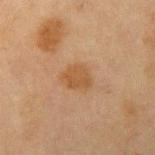workup: total-body-photography surveillance lesion; no biopsy
lesion size: ≈3 mm
image source: total-body-photography crop, ~15 mm field of view
subject: male, in their mid- to late 60s
TBP lesion metrics: an area of roughly 6 mm²; a border-irregularity index near 2/10 and a peripheral color-asymmetry measure near 1
anatomic site: the left upper arm